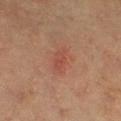A female patient, aged 53 to 57. A 15 mm close-up extracted from a 3D total-body photography capture. On the left lower leg.